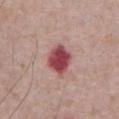notes: imaged on a skin check; not biopsied | subject: male, in their mid- to late 60s | TBP lesion metrics: an automated nevus-likeness rating near 0 out of 100 and a lesion-detection confidence of about 100/100 | body site: the abdomen | image source: total-body-photography crop, ~15 mm field of view.